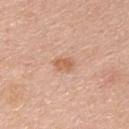| key | value |
|---|---|
| biopsy status | catalogued during a skin exam; not biopsied |